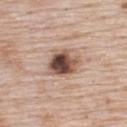Part of a total-body skin-imaging series; this lesion was reviewed on a skin check and was not flagged for biopsy. The patient is a female aged around 70. On the upper back. This is a white-light tile. Automated image analysis of the tile measured a lesion area of about 10 mm² and an eccentricity of roughly 0.6. It also reported an average lesion color of about L≈50 a*≈18 b*≈25 (CIELAB) and roughly 19 lightness units darker than nearby skin. About 4 mm across. A 15 mm close-up extracted from a 3D total-body photography capture.